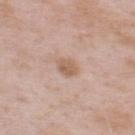The lesion was tiled from a total-body skin photograph and was not biopsied. The subject is a male approximately 55 years of age. Captured under white-light illumination. Cropped from a total-body skin-imaging series; the visible field is about 15 mm. Automated image analysis of the tile measured a lesion color around L≈59 a*≈18 b*≈29 in CIELAB and a lesion-to-skin contrast of about 7 (normalized; higher = more distinct). The software also gave a border-irregularity rating of about 2/10, a within-lesion color-variation index near 2/10, and peripheral color asymmetry of about 1. It also reported a nevus-likeness score of about 15/100 and a lesion-detection confidence of about 100/100. The lesion is located on the upper back.